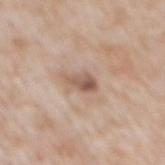Assessment:
Part of a total-body skin-imaging series; this lesion was reviewed on a skin check and was not flagged for biopsy.
Image and clinical context:
Captured under white-light illumination. The lesion is located on the mid back. A male subject aged 48 to 52. A 15 mm crop from a total-body photograph taken for skin-cancer surveillance. The recorded lesion diameter is about 3 mm.